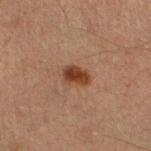Assessment: This lesion was catalogued during total-body skin photography and was not selected for biopsy. Background: This image is a 15 mm lesion crop taken from a total-body photograph. This is a cross-polarized tile. The lesion is located on the leg. A male subject approximately 30 years of age.